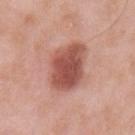Assessment: Imaged during a routine full-body skin examination; the lesion was not biopsied and no histopathology is available. Background: The lesion is on the left upper arm. A male subject aged around 55. Captured under white-light illumination. Cropped from a whole-body photographic skin survey; the tile spans about 15 mm. The lesion's longest dimension is about 5.5 mm.